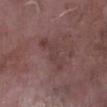Findings:
– follow-up · total-body-photography surveillance lesion; no biopsy
– lighting · white-light
– lesion diameter · ≈5.5 mm
– image · ~15 mm tile from a whole-body skin photo
– patient · male, aged 73–77
– body site · the right lower leg